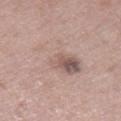Case summary:
* workup: total-body-photography surveillance lesion; no biopsy
* location: the right thigh
* imaging modality: 15 mm crop, total-body photography
* subject: female, about 75 years old